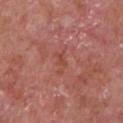Q: Was a biopsy performed?
A: imaged on a skin check; not biopsied